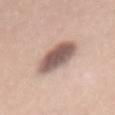notes = imaged on a skin check; not biopsied | location = the mid back | patient = female, aged 43–47 | image source = ~15 mm crop, total-body skin-cancer survey.